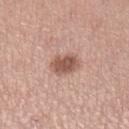The lesion was tiled from a total-body skin photograph and was not biopsied.
From the left lower leg.
The patient is a female approximately 45 years of age.
A 15 mm close-up tile from a total-body photography series done for melanoma screening.